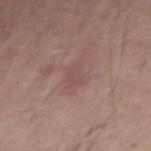  biopsy_status: not biopsied; imaged during a skin examination
  lesion_size:
    long_diameter_mm_approx: 3.0
  image:
    source: total-body photography crop
    field_of_view_mm: 15
  automated_metrics:
    cielab_L: 49
    cielab_a: 21
    cielab_b: 18
    border_irregularity_0_10: 3.0
    color_variation_0_10: 1.0
    nevus_likeness_0_100: 0
  patient:
    sex: male
    age_approx: 55
  lighting: white-light